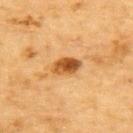<lesion>
  <biopsy_status>not biopsied; imaged during a skin examination</biopsy_status>
  <site>upper back</site>
  <lesion_size>
    <long_diameter_mm_approx>3.5</long_diameter_mm_approx>
  </lesion_size>
  <image>
    <source>total-body photography crop</source>
    <field_of_view_mm>15</field_of_view_mm>
  </image>
  <patient>
    <sex>male</sex>
    <age_approx>85+</age_approx>
  </patient>
</lesion>